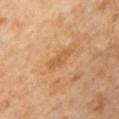The lesion was tiled from a total-body skin photograph and was not biopsied. The lesion is on the chest. The lesion's longest dimension is about 3.5 mm. A male patient aged around 60. An algorithmic analysis of the crop reported an outline eccentricity of about 0.95 (0 = round, 1 = elongated) and a shape-asymmetry score of about 0.3 (0 = symmetric). The analysis additionally found a border-irregularity rating of about 4/10 and a color-variation rating of about 1.5/10. And it measured an automated nevus-likeness rating near 0 out of 100. A roughly 15 mm field-of-view crop from a total-body skin photograph.